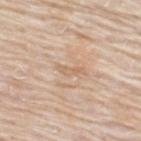Assessment: The lesion was tiled from a total-body skin photograph and was not biopsied. Context: From the back. Longest diameter approximately 3 mm. A male patient, in their mid-70s. A lesion tile, about 15 mm wide, cut from a 3D total-body photograph.